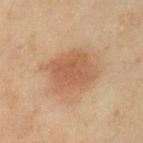<case>
  <biopsy_status>not biopsied; imaged during a skin examination</biopsy_status>
  <automated_metrics>
    <area_mm2_approx>19.0</area_mm2_approx>
    <shape_asymmetry>0.15</shape_asymmetry>
    <cielab_L>50</cielab_L>
    <cielab_a>19</cielab_a>
    <cielab_b>30</cielab_b>
    <vs_skin_darker_L>9.0</vs_skin_darker_L>
    <nevus_likeness_0_100>95</nevus_likeness_0_100>
    <lesion_detection_confidence_0_100>100</lesion_detection_confidence_0_100>
  </automated_metrics>
  <patient>
    <sex>female</sex>
    <age_approx>55</age_approx>
  </patient>
  <lighting>cross-polarized</lighting>
  <image>
    <source>total-body photography crop</source>
    <field_of_view_mm>15</field_of_view_mm>
  </image>
  <site>leg</site>
  <lesion_size>
    <long_diameter_mm_approx>6.0</long_diameter_mm_approx>
  </lesion_size>
</case>